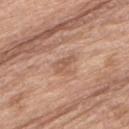The lesion was photographed on a routine skin check and not biopsied; there is no pathology result. A 15 mm close-up tile from a total-body photography series done for melanoma screening. Imaged with white-light lighting. Longest diameter approximately 3 mm. A male patient, aged approximately 70. The lesion is on the lower back.